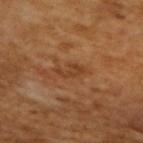follow-up = catalogued during a skin exam; not biopsied | automated metrics = a shape eccentricity near 0.9 and a symmetry-axis asymmetry near 0.4; a border-irregularity rating of about 5.5/10 and a within-lesion color-variation index near 0/10; a nevus-likeness score of about 0/100 and lesion-presence confidence of about 100/100 | acquisition = total-body-photography crop, ~15 mm field of view | patient = male, aged approximately 65 | lesion diameter = ≈3 mm | tile lighting = cross-polarized illumination.